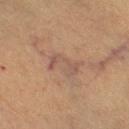<tbp_lesion>
  <biopsy_status>not biopsied; imaged during a skin examination</biopsy_status>
  <patient>
    <sex>female</sex>
    <age_approx>40</age_approx>
  </patient>
  <automated_metrics>
    <cielab_L>45</cielab_L>
    <cielab_a>16</cielab_a>
    <cielab_b>23</cielab_b>
    <vs_skin_darker_L>6.0</vs_skin_darker_L>
    <vs_skin_contrast_norm>5.5</vs_skin_contrast_norm>
  </automated_metrics>
  <site>leg</site>
  <lighting>cross-polarized</lighting>
  <lesion_size>
    <long_diameter_mm_approx>4.0</long_diameter_mm_approx>
  </lesion_size>
  <image>
    <source>total-body photography crop</source>
    <field_of_view_mm>15</field_of_view_mm>
  </image>
</tbp_lesion>